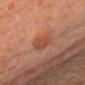Q: What is the lesion's diameter?
A: ≈3 mm
Q: Where on the body is the lesion?
A: the front of the torso
Q: What lighting was used for the tile?
A: cross-polarized
Q: What is the imaging modality?
A: ~15 mm tile from a whole-body skin photo
Q: What did automated image analysis measure?
A: an average lesion color of about L≈50 a*≈28 b*≈33 (CIELAB) and a normalized lesion–skin contrast near 6; a nevus-likeness score of about 100/100 and a detector confidence of about 100 out of 100 that the crop contains a lesion
Q: Patient demographics?
A: female, about 55 years old